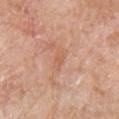Q: Was a biopsy performed?
A: total-body-photography surveillance lesion; no biopsy
Q: What are the patient's age and sex?
A: female, approximately 75 years of age
Q: What did automated image analysis measure?
A: a mean CIELAB color near L≈60 a*≈23 b*≈34, a lesion–skin lightness drop of about 6, and a lesion-to-skin contrast of about 5 (normalized; higher = more distinct); an automated nevus-likeness rating near 0 out of 100 and lesion-presence confidence of about 100/100
Q: Lesion size?
A: ~2.5 mm (longest diameter)
Q: What kind of image is this?
A: ~15 mm crop, total-body skin-cancer survey
Q: Where on the body is the lesion?
A: the front of the torso